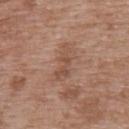Imaged during a routine full-body skin examination; the lesion was not biopsied and no histopathology is available.
Located on the upper back.
This is a white-light tile.
A male patient aged around 50.
The total-body-photography lesion software estimated a lesion color around L≈50 a*≈20 b*≈28 in CIELAB and roughly 8 lightness units darker than nearby skin.
The lesion's longest dimension is about 4.5 mm.
A roughly 15 mm field-of-view crop from a total-body skin photograph.